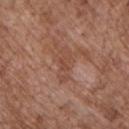notes: no biopsy performed (imaged during a skin exam) | imaging modality: ~15 mm crop, total-body skin-cancer survey | automated metrics: an average lesion color of about L≈47 a*≈22 b*≈28 (CIELAB) and roughly 6 lightness units darker than nearby skin; a border-irregularity index near 5/10, a color-variation rating of about 2/10, and peripheral color asymmetry of about 0.5 | anatomic site: the chest | diameter: ~4.5 mm (longest diameter) | tile lighting: white-light illumination | patient: male, aged 68 to 72.